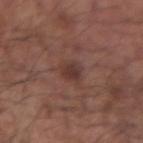| field | value |
|---|---|
| follow-up | imaged on a skin check; not biopsied |
| patient | male, aged 53–57 |
| lesion diameter | ~2.5 mm (longest diameter) |
| tile lighting | white-light |
| imaging modality | ~15 mm tile from a whole-body skin photo |
| body site | the left forearm |